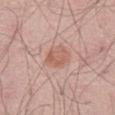Captured during whole-body skin photography for melanoma surveillance; the lesion was not biopsied. Longest diameter approximately 3.5 mm. Captured under white-light illumination. Cropped from a total-body skin-imaging series; the visible field is about 15 mm. A male patient aged 58–62. Automated tile analysis of the lesion measured a border-irregularity index near 2/10, internal color variation of about 3 on a 0–10 scale, and a peripheral color-asymmetry measure near 1. The lesion is on the abdomen.